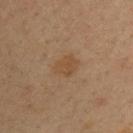biopsy status=catalogued during a skin exam; not biopsied
subject=male, in their mid-30s
image source=total-body-photography crop, ~15 mm field of view
location=the front of the torso
tile lighting=cross-polarized illumination
lesion size=about 3 mm